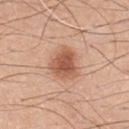This lesion was catalogued during total-body skin photography and was not selected for biopsy.
A male subject, aged approximately 50.
Automated image analysis of the tile measured peripheral color asymmetry of about 1.
The lesion is on the front of the torso.
About 4 mm across.
A 15 mm crop from a total-body photograph taken for skin-cancer surveillance.